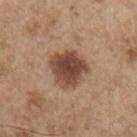Clinical impression:
Imaged during a routine full-body skin examination; the lesion was not biopsied and no histopathology is available.
Clinical summary:
From the chest. The subject is a male about 55 years old. Approximately 5 mm at its widest. A 15 mm close-up tile from a total-body photography series done for melanoma screening.